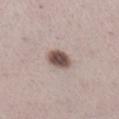  biopsy_status: not biopsied; imaged during a skin examination
  site: right lower leg
  image:
    source: total-body photography crop
    field_of_view_mm: 15
  patient:
    sex: female
    age_approx: 40
  lighting: white-light
  lesion_size:
    long_diameter_mm_approx: 3.5